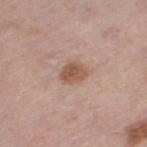Q: Was a biopsy performed?
A: no biopsy performed (imaged during a skin exam)
Q: Patient demographics?
A: female, aged 63 to 67
Q: What is the anatomic site?
A: the left thigh
Q: How was this image acquired?
A: ~15 mm crop, total-body skin-cancer survey